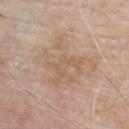This lesion was catalogued during total-body skin photography and was not selected for biopsy. Cropped from a whole-body photographic skin survey; the tile spans about 15 mm. The total-body-photography lesion software estimated an area of roughly 28 mm², a shape eccentricity near 0.45, and a symmetry-axis asymmetry near 0.4. The analysis additionally found an average lesion color of about L≈61 a*≈15 b*≈29 (CIELAB) and a lesion–skin lightness drop of about 6. And it measured a detector confidence of about 100 out of 100 that the crop contains a lesion. On the chest. The lesion's longest dimension is about 6.5 mm. Imaged with white-light lighting. A male patient aged approximately 80.